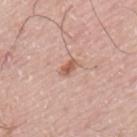Q: Is there a histopathology result?
A: catalogued during a skin exam; not biopsied
Q: Patient demographics?
A: male, aged 73–77
Q: What is the lesion's diameter?
A: ~2.5 mm (longest diameter)
Q: What is the anatomic site?
A: the mid back
Q: What kind of image is this?
A: 15 mm crop, total-body photography
Q: Illumination type?
A: white-light